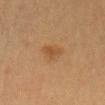Clinical summary: The tile uses cross-polarized illumination. On the lower back. Automated tile analysis of the lesion measured a lesion area of about 5 mm² and a symmetry-axis asymmetry near 0.35. And it measured a lesion color around L≈41 a*≈17 b*≈32 in CIELAB and a normalized border contrast of about 6. About 3 mm across. A female subject, aged around 40. A region of skin cropped from a whole-body photographic capture, roughly 15 mm wide.